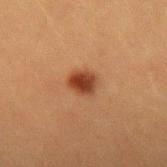biopsy status=no biopsy performed (imaged during a skin exam)
site=the arm
patient=male, aged around 40
image=15 mm crop, total-body photography